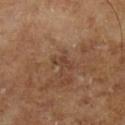diameter — ~3 mm (longest diameter)
subject — male, roughly 65 years of age
imaging modality — ~15 mm tile from a whole-body skin photo
image-analysis metrics — an outline eccentricity of about 0.9 (0 = round, 1 = elongated) and a shape-asymmetry score of about 0.4 (0 = symmetric); border irregularity of about 4.5 on a 0–10 scale, a color-variation rating of about 0/10, and radial color variation of about 0; a classifier nevus-likeness of about 0/100 and a detector confidence of about 100 out of 100 that the crop contains a lesion